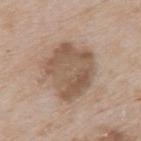Q: Was this lesion biopsied?
A: catalogued during a skin exam; not biopsied
Q: What are the patient's age and sex?
A: male, aged around 65
Q: What is the imaging modality?
A: 15 mm crop, total-body photography
Q: Where on the body is the lesion?
A: the mid back